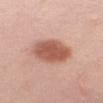Assessment: The lesion was photographed on a routine skin check and not biopsied; there is no pathology result. Acquisition and patient details: A female subject aged around 40. On the front of the torso. A close-up tile cropped from a whole-body skin photograph, about 15 mm across.